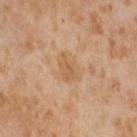Imaged during a routine full-body skin examination; the lesion was not biopsied and no histopathology is available. A female subject in their mid- to late 50s. From the right thigh. A 15 mm close-up extracted from a 3D total-body photography capture. About 3.5 mm across.